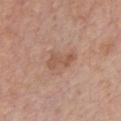* workup: no biopsy performed (imaged during a skin exam)
* lighting: white-light
* image source: ~15 mm crop, total-body skin-cancer survey
* lesion size: ~3.5 mm (longest diameter)
* patient: male, in their 60s
* anatomic site: the chest